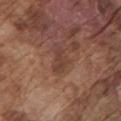| feature | finding |
|---|---|
| imaging modality | ~15 mm tile from a whole-body skin photo |
| diameter | ≈3.5 mm |
| site | the left upper arm |
| subject | male, in their mid- to late 70s |
| lighting | white-light illumination |